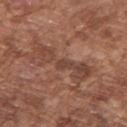Findings:
– follow-up — total-body-photography surveillance lesion; no biopsy
– tile lighting — white-light illumination
– image — 15 mm crop, total-body photography
– anatomic site — the right upper arm
– TBP lesion metrics — an area of roughly 12 mm² and an eccentricity of roughly 0.95
– subject — male, approximately 75 years of age